The patient is a male about 45 years old; from the upper back; a 15 mm crop from a total-body photograph taken for skin-cancer surveillance:
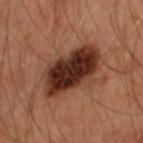| field | value |
|---|---|
| image-analysis metrics | an average lesion color of about L≈33 a*≈23 b*≈28 (CIELAB), roughly 17 lightness units darker than nearby skin, and a normalized lesion–skin contrast near 14; border irregularity of about 2.5 on a 0–10 scale and peripheral color asymmetry of about 2.5; a classifier nevus-likeness of about 0/100 and a detector confidence of about 100 out of 100 that the crop contains a lesion |
| lighting | cross-polarized |
| size | ~7.5 mm (longest diameter) |
| histopathology | an atypical melanocytic neoplasm (borderline) |